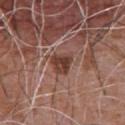The lesion was tiled from a total-body skin photograph and was not biopsied. An algorithmic analysis of the crop reported an outline eccentricity of about 0.55 (0 = round, 1 = elongated) and a symmetry-axis asymmetry near 0.3. The analysis additionally found a mean CIELAB color near L≈41 a*≈21 b*≈25, about 12 CIELAB-L* units darker than the surrounding skin, and a normalized lesion–skin contrast near 9.5. The analysis additionally found a border-irregularity index near 2.5/10, internal color variation of about 4 on a 0–10 scale, and peripheral color asymmetry of about 1.5. Captured under white-light illumination. Longest diameter approximately 3 mm. The lesion is on the chest. The patient is a male aged approximately 75. A 15 mm crop from a total-body photograph taken for skin-cancer surveillance.